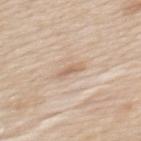This lesion was catalogued during total-body skin photography and was not selected for biopsy.
On the mid back.
Cropped from a whole-body photographic skin survey; the tile spans about 15 mm.
A male patient, aged 58–62.